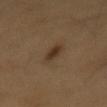Case summary:
* biopsy status — no biopsy performed (imaged during a skin exam)
* lighting — cross-polarized illumination
* lesion size — ≈3.5 mm
* imaging modality — ~15 mm tile from a whole-body skin photo
* patient — male, roughly 60 years of age
* anatomic site — the mid back
* TBP lesion metrics — a lesion color around L≈33 a*≈14 b*≈26 in CIELAB, about 9 CIELAB-L* units darker than the surrounding skin, and a lesion-to-skin contrast of about 8.5 (normalized; higher = more distinct); a border-irregularity rating of about 3.5/10 and a within-lesion color-variation index near 2.5/10; a nevus-likeness score of about 100/100